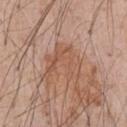Recorded during total-body skin imaging; not selected for excision or biopsy.
This is a white-light tile.
A male patient, aged 53–57.
The total-body-photography lesion software estimated an average lesion color of about L≈55 a*≈21 b*≈30 (CIELAB) and about 7 CIELAB-L* units darker than the surrounding skin. And it measured a lesion-detection confidence of about 90/100.
The lesion is on the front of the torso.
A 15 mm close-up extracted from a 3D total-body photography capture.
The recorded lesion diameter is about 5 mm.